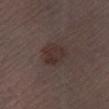Q: Is there a histopathology result?
A: no biopsy performed (imaged during a skin exam)
Q: What is the anatomic site?
A: the left lower leg
Q: Automated lesion metrics?
A: a lesion color around L≈30 a*≈14 b*≈17 in CIELAB, a lesion–skin lightness drop of about 6, and a normalized border contrast of about 7
Q: What kind of image is this?
A: total-body-photography crop, ~15 mm field of view
Q: Patient demographics?
A: male, aged 48 to 52
Q: What lighting was used for the tile?
A: white-light illumination